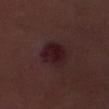Q: Was this lesion biopsied?
A: no biopsy performed (imaged during a skin exam)
Q: How large is the lesion?
A: ~3.5 mm (longest diameter)
Q: What kind of image is this?
A: ~15 mm crop, total-body skin-cancer survey
Q: What are the patient's age and sex?
A: female, roughly 55 years of age
Q: Where on the body is the lesion?
A: the abdomen
Q: How was the tile lit?
A: cross-polarized
Q: What did automated image analysis measure?
A: a lesion-to-skin contrast of about 10.5 (normalized; higher = more distinct); internal color variation of about 4.5 on a 0–10 scale and peripheral color asymmetry of about 1.5